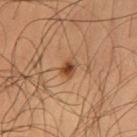Assessment:
Part of a total-body skin-imaging series; this lesion was reviewed on a skin check and was not flagged for biopsy.
Image and clinical context:
Imaged with cross-polarized lighting. The lesion is located on the left thigh. The total-body-photography lesion software estimated a shape eccentricity near 0.75. The analysis additionally found a lesion color around L≈46 a*≈23 b*≈34 in CIELAB and a lesion-to-skin contrast of about 8.5 (normalized; higher = more distinct). The lesion's longest dimension is about 2.5 mm. A male patient approximately 60 years of age. A 15 mm close-up extracted from a 3D total-body photography capture.